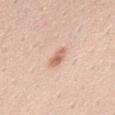A male patient, aged around 50. Imaged with white-light lighting. A 15 mm close-up extracted from a 3D total-body photography capture. On the mid back. Automated tile analysis of the lesion measured an area of roughly 3.5 mm², an eccentricity of roughly 0.9, and two-axis asymmetry of about 0.2. Approximately 3 mm at its widest.